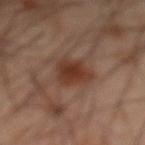A lesion tile, about 15 mm wide, cut from a 3D total-body photograph. A male patient, aged 63 to 67. From the abdomen.